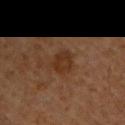{
  "biopsy_status": "not biopsied; imaged during a skin examination",
  "image": {
    "source": "total-body photography crop",
    "field_of_view_mm": 15
  },
  "patient": {
    "sex": "male",
    "age_approx": 60
  },
  "site": "right upper arm"
}